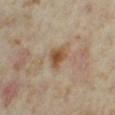follow-up: no biopsy performed (imaged during a skin exam)
lesion diameter: about 2.5 mm
subject: female, aged 33–37
image source: ~15 mm crop, total-body skin-cancer survey
anatomic site: the left thigh